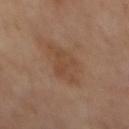The lesion was tiled from a total-body skin photograph and was not biopsied. About 5.5 mm across. This image is a 15 mm lesion crop taken from a total-body photograph. From the mid back.